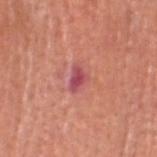Q: Was a biopsy performed?
A: imaged on a skin check; not biopsied
Q: Where on the body is the lesion?
A: the head or neck
Q: How was this image acquired?
A: 15 mm crop, total-body photography
Q: What are the patient's age and sex?
A: male, aged 63 to 67